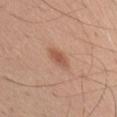This lesion was catalogued during total-body skin photography and was not selected for biopsy. A male patient in their mid- to late 50s. The lesion is located on the right upper arm. About 3 mm across. The tile uses white-light illumination. A 15 mm close-up extracted from a 3D total-body photography capture.